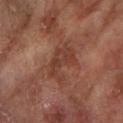Notes:
– follow-up — no biopsy performed (imaged during a skin exam)
– subject — male, aged 83 or older
– illumination — cross-polarized
– anatomic site — the left forearm
– automated metrics — a mean CIELAB color near L≈37 a*≈23 b*≈26, about 7 CIELAB-L* units darker than the surrounding skin, and a normalized lesion–skin contrast near 6
– lesion diameter — ≈4.5 mm
– image — 15 mm crop, total-body photography